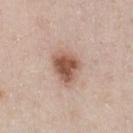Assessment: Part of a total-body skin-imaging series; this lesion was reviewed on a skin check and was not flagged for biopsy. Acquisition and patient details: The lesion's longest dimension is about 4 mm. From the chest. The lesion-visualizer software estimated a footprint of about 10 mm² and a shape eccentricity near 0.6. The software also gave a classifier nevus-likeness of about 95/100 and a detector confidence of about 100 out of 100 that the crop contains a lesion. Captured under white-light illumination. A region of skin cropped from a whole-body photographic capture, roughly 15 mm wide. A male subject in their 40s.